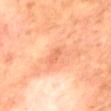This lesion was catalogued during total-body skin photography and was not selected for biopsy. A close-up tile cropped from a whole-body skin photograph, about 15 mm across. A male patient aged around 70. On the mid back.